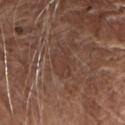Clinical impression: Recorded during total-body skin imaging; not selected for excision or biopsy. Background: A 15 mm close-up extracted from a 3D total-body photography capture. A male patient in their mid-70s. The lesion is on the front of the torso. The recorded lesion diameter is about 3.5 mm.